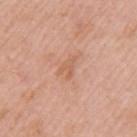{"biopsy_status": "not biopsied; imaged during a skin examination", "site": "left upper arm", "lesion_size": {"long_diameter_mm_approx": 2.5}, "patient": {"sex": "female", "age_approx": 40}, "image": {"source": "total-body photography crop", "field_of_view_mm": 15}}